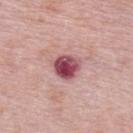Imaged during a routine full-body skin examination; the lesion was not biopsied and no histopathology is available.